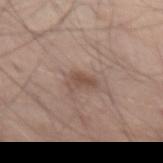biopsy status — no biopsy performed (imaged during a skin exam)
imaging modality — ~15 mm crop, total-body skin-cancer survey
TBP lesion metrics — a lesion area of about 4.5 mm², an outline eccentricity of about 0.85 (0 = round, 1 = elongated), and a shape-asymmetry score of about 0.35 (0 = symmetric); a lesion color around L≈50 a*≈17 b*≈25 in CIELAB, about 9 CIELAB-L* units darker than the surrounding skin, and a normalized border contrast of about 6.5; a border-irregularity rating of about 3.5/10, a within-lesion color-variation index near 3/10, and peripheral color asymmetry of about 1; a nevus-likeness score of about 35/100 and lesion-presence confidence of about 100/100
body site — the abdomen
tile lighting — white-light
diameter — ≈3 mm
patient — male, aged 63 to 67